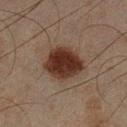Captured during whole-body skin photography for melanoma surveillance; the lesion was not biopsied. The lesion is located on the left lower leg. This is a cross-polarized tile. The lesion's longest dimension is about 5 mm. A male subject, in their mid- to late 40s. A 15 mm close-up extracted from a 3D total-body photography capture.